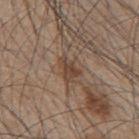Captured during whole-body skin photography for melanoma surveillance; the lesion was not biopsied.
The recorded lesion diameter is about 3 mm.
An algorithmic analysis of the crop reported a footprint of about 4.5 mm², an eccentricity of roughly 0.75, and two-axis asymmetry of about 0.45. And it measured a classifier nevus-likeness of about 0/100 and lesion-presence confidence of about 95/100.
The lesion is on the mid back.
Captured under white-light illumination.
A lesion tile, about 15 mm wide, cut from a 3D total-body photograph.
A male subject, aged around 45.